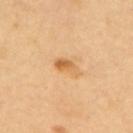Clinical impression:
This lesion was catalogued during total-body skin photography and was not selected for biopsy.
Background:
A lesion tile, about 15 mm wide, cut from a 3D total-body photograph. Captured under cross-polarized illumination. A female subject aged 58 to 62. On the upper back. An algorithmic analysis of the crop reported a classifier nevus-likeness of about 60/100. The lesion's longest dimension is about 3.5 mm.